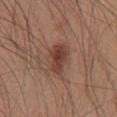Clinical impression: This lesion was catalogued during total-body skin photography and was not selected for biopsy. Clinical summary: On the chest. A lesion tile, about 15 mm wide, cut from a 3D total-body photograph. The tile uses white-light illumination. Measured at roughly 4.5 mm in maximum diameter. The subject is a male aged around 60.